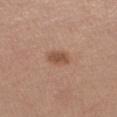biopsy status: imaged on a skin check; not biopsied
lighting: white-light illumination
anatomic site: the left thigh
TBP lesion metrics: an average lesion color of about L≈51 a*≈21 b*≈31 (CIELAB), roughly 10 lightness units darker than nearby skin, and a lesion-to-skin contrast of about 7.5 (normalized; higher = more distinct); an automated nevus-likeness rating near 90 out of 100 and lesion-presence confidence of about 100/100
patient: female, aged 63–67
diameter: ~2.5 mm (longest diameter)
imaging modality: total-body-photography crop, ~15 mm field of view